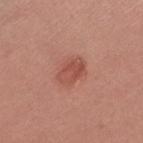Q: Is there a histopathology result?
A: no biopsy performed (imaged during a skin exam)
Q: Where on the body is the lesion?
A: the left upper arm
Q: How was this image acquired?
A: 15 mm crop, total-body photography
Q: Who is the patient?
A: female, aged around 35